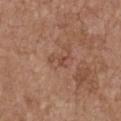Q: Was this lesion biopsied?
A: imaged on a skin check; not biopsied
Q: Patient demographics?
A: female, aged around 65
Q: Automated lesion metrics?
A: an outline eccentricity of about 0.85 (0 = round, 1 = elongated) and a shape-asymmetry score of about 0.5 (0 = symmetric)
Q: What is the anatomic site?
A: the chest
Q: Lesion size?
A: ≈3 mm
Q: What is the imaging modality?
A: ~15 mm tile from a whole-body skin photo
Q: What lighting was used for the tile?
A: white-light